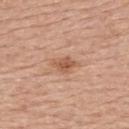Q: Is there a histopathology result?
A: no biopsy performed (imaged during a skin exam)
Q: What kind of image is this?
A: 15 mm crop, total-body photography
Q: Illumination type?
A: white-light
Q: Lesion location?
A: the upper back
Q: Who is the patient?
A: female, roughly 65 years of age
Q: Lesion size?
A: about 3.5 mm